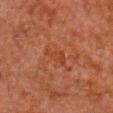Clinical impression: This lesion was catalogued during total-body skin photography and was not selected for biopsy. Acquisition and patient details: About 3.5 mm across. The total-body-photography lesion software estimated a classifier nevus-likeness of about 0/100 and a detector confidence of about 100 out of 100 that the crop contains a lesion. A roughly 15 mm field-of-view crop from a total-body skin photograph. From the front of the torso. Captured under cross-polarized illumination. A male patient aged 78 to 82.